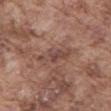{
  "biopsy_status": "not biopsied; imaged during a skin examination",
  "site": "mid back",
  "patient": {
    "sex": "male",
    "age_approx": 75
  },
  "image": {
    "source": "total-body photography crop",
    "field_of_view_mm": 15
  },
  "lesion_size": {
    "long_diameter_mm_approx": 4.5
  }
}